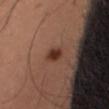This lesion was catalogued during total-body skin photography and was not selected for biopsy. This is a cross-polarized tile. Located on the right thigh. A close-up tile cropped from a whole-body skin photograph, about 15 mm across. The patient is a male aged 58 to 62.